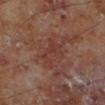{
  "biopsy_status": "not biopsied; imaged during a skin examination",
  "lesion_size": {
    "long_diameter_mm_approx": 4.0
  },
  "lighting": "cross-polarized",
  "image": {
    "source": "total-body photography crop",
    "field_of_view_mm": 15
  },
  "patient": {
    "sex": "male",
    "age_approx": 70
  },
  "site": "left lower leg"
}